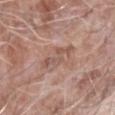Automated image analysis of the tile measured a lesion area of about 5.5 mm², a shape eccentricity near 0.85, and a shape-asymmetry score of about 0.4 (0 = symmetric). It also reported border irregularity of about 8 on a 0–10 scale and a within-lesion color-variation index near 2/10. The software also gave a nevus-likeness score of about 0/100. Cropped from a whole-body photographic skin survey; the tile spans about 15 mm. The patient is a male about 75 years old. This is a white-light tile. About 3.5 mm across. The lesion is on the right forearm.